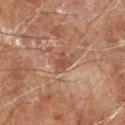biopsy status = imaged on a skin check; not biopsied
subject = male, in their 60s
body site = the left forearm
size = ~3 mm (longest diameter)
image source = ~15 mm crop, total-body skin-cancer survey